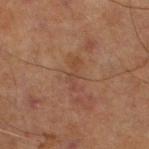<record>
  <biopsy_status>not biopsied; imaged during a skin examination</biopsy_status>
  <lesion_size>
    <long_diameter_mm_approx>4.0</long_diameter_mm_approx>
  </lesion_size>
  <automated_metrics>
    <eccentricity>0.95</eccentricity>
    <shape_asymmetry>0.4</shape_asymmetry>
    <border_irregularity_0_10>6.0</border_irregularity_0_10>
    <color_variation_0_10>1.0</color_variation_0_10>
    <peripheral_color_asymmetry>0.0</peripheral_color_asymmetry>
  </automated_metrics>
  <site>left lower leg</site>
  <patient>
    <sex>male</sex>
    <age_approx>70</age_approx>
  </patient>
  <image>
    <source>total-body photography crop</source>
    <field_of_view_mm>15</field_of_view_mm>
  </image>
</record>